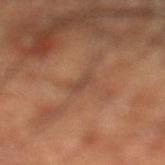workup: no biopsy performed (imaged during a skin exam)
imaging modality: ~15 mm tile from a whole-body skin photo
patient: male, aged around 60
image-analysis metrics: about 6 CIELAB-L* units darker than the surrounding skin and a lesion-to-skin contrast of about 5.5 (normalized; higher = more distinct); a nevus-likeness score of about 0/100 and a lesion-detection confidence of about 60/100
anatomic site: the left lower leg